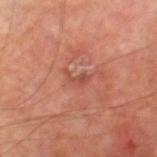Assessment: No biopsy was performed on this lesion — it was imaged during a full skin examination and was not determined to be concerning. Image and clinical context: Imaged with cross-polarized lighting. This image is a 15 mm lesion crop taken from a total-body photograph. About 2.5 mm across. The lesion-visualizer software estimated a mean CIELAB color near L≈48 a*≈28 b*≈29. The patient is a male aged 68–72. Located on the right thigh.